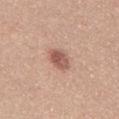biopsy status: total-body-photography surveillance lesion; no biopsy
anatomic site: the mid back
patient: male, approximately 25 years of age
size: ~3.5 mm (longest diameter)
acquisition: 15 mm crop, total-body photography
tile lighting: white-light